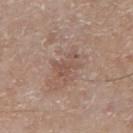Impression:
Imaged during a routine full-body skin examination; the lesion was not biopsied and no histopathology is available.
Clinical summary:
A male subject, aged 63 to 67. Automated image analysis of the tile measured an average lesion color of about L≈52 a*≈18 b*≈25 (CIELAB), roughly 7 lightness units darker than nearby skin, and a lesion-to-skin contrast of about 5 (normalized; higher = more distinct). The analysis additionally found a border-irregularity rating of about 7/10, a color-variation rating of about 1/10, and peripheral color asymmetry of about 0.5. A lesion tile, about 15 mm wide, cut from a 3D total-body photograph. On the right lower leg. Longest diameter approximately 3.5 mm. Imaged with white-light lighting.